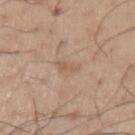A 15 mm close-up tile from a total-body photography series done for melanoma screening.
From the arm.
A male patient, in their mid- to late 50s.